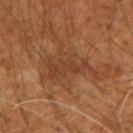Recorded during total-body skin imaging; not selected for excision or biopsy. Automated image analysis of the tile measured a footprint of about 14 mm², a shape eccentricity near 0.75, and a shape-asymmetry score of about 0.4 (0 = symmetric). The analysis additionally found a lesion color around L≈38 a*≈21 b*≈31 in CIELAB, a lesion–skin lightness drop of about 6, and a normalized border contrast of about 5.5. From the right upper arm. Captured under cross-polarized illumination. A male patient, aged 58 to 62. A close-up tile cropped from a whole-body skin photograph, about 15 mm across.